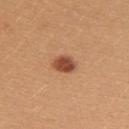Recorded during total-body skin imaging; not selected for excision or biopsy. A female patient in their mid- to late 20s. A 15 mm close-up tile from a total-body photography series done for melanoma screening. Automated tile analysis of the lesion measured an average lesion color of about L≈49 a*≈26 b*≈34 (CIELAB), about 15 CIELAB-L* units darker than the surrounding skin, and a normalized lesion–skin contrast near 10.5. The software also gave a within-lesion color-variation index near 4/10 and radial color variation of about 1.5. The software also gave a nevus-likeness score of about 100/100 and a detector confidence of about 100 out of 100 that the crop contains a lesion. On the arm. The recorded lesion diameter is about 3 mm. This is a white-light tile.